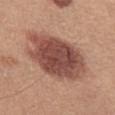Assessment: Imaged during a routine full-body skin examination; the lesion was not biopsied and no histopathology is available. Image and clinical context: Automated tile analysis of the lesion measured a footprint of about 33 mm², a shape eccentricity near 0.75, and two-axis asymmetry of about 0.15. The software also gave an average lesion color of about L≈48 a*≈23 b*≈26 (CIELAB), a lesion–skin lightness drop of about 15, and a lesion-to-skin contrast of about 10.5 (normalized; higher = more distinct). This is a white-light tile. Cropped from a total-body skin-imaging series; the visible field is about 15 mm. A female subject approximately 55 years of age. The recorded lesion diameter is about 8.5 mm. From the left thigh.